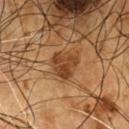<tbp_lesion>
<biopsy_status>not biopsied; imaged during a skin examination</biopsy_status>
<lesion_size>
  <long_diameter_mm_approx>3.5</long_diameter_mm_approx>
</lesion_size>
<image>
  <source>total-body photography crop</source>
  <field_of_view_mm>15</field_of_view_mm>
</image>
<patient>
  <sex>male</sex>
  <age_approx>55</age_approx>
</patient>
<site>chest</site>
<lighting>cross-polarized</lighting>
</tbp_lesion>